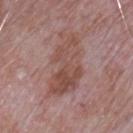This lesion was catalogued during total-body skin photography and was not selected for biopsy.
A male subject aged 63–67.
This is a white-light tile.
The lesion is on the front of the torso.
A 15 mm close-up extracted from a 3D total-body photography capture.